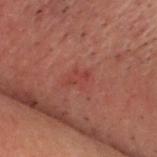Part of a total-body skin-imaging series; this lesion was reviewed on a skin check and was not flagged for biopsy.
Longest diameter approximately 2.5 mm.
The lesion-visualizer software estimated a border-irregularity index near 3/10, a within-lesion color-variation index near 0.5/10, and radial color variation of about 0.5. The software also gave a nevus-likeness score of about 0/100 and lesion-presence confidence of about 100/100.
The lesion is on the head or neck.
The tile uses cross-polarized illumination.
A male subject aged 68 to 72.
A 15 mm crop from a total-body photograph taken for skin-cancer surveillance.